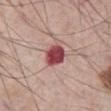notes: no biopsy performed (imaged during a skin exam); image source: ~15 mm tile from a whole-body skin photo; lesion size: about 3.5 mm; patient: male, in their mid- to late 70s; body site: the abdomen.